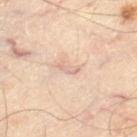workup = total-body-photography surveillance lesion; no biopsy
image = ~15 mm crop, total-body skin-cancer survey
subject = approximately 55 years of age
location = the left thigh